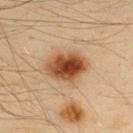Assessment: No biopsy was performed on this lesion — it was imaged during a full skin examination and was not determined to be concerning. Image and clinical context: A region of skin cropped from a whole-body photographic capture, roughly 15 mm wide. The lesion's longest dimension is about 5 mm. The subject is a male aged 33–37. The lesion is on the upper back. This is a cross-polarized tile.